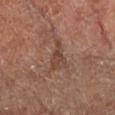The lesion was photographed on a routine skin check and not biopsied; there is no pathology result. Cropped from a whole-body photographic skin survey; the tile spans about 15 mm. The patient is aged 63–67. An algorithmic analysis of the crop reported an area of roughly 5 mm², an eccentricity of roughly 0.85, and a shape-asymmetry score of about 0.55 (0 = symmetric). And it measured a nevus-likeness score of about 0/100 and lesion-presence confidence of about 85/100. The lesion is on the right lower leg. Imaged with cross-polarized lighting. The lesion's longest dimension is about 3.5 mm.